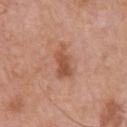Part of a total-body skin-imaging series; this lesion was reviewed on a skin check and was not flagged for biopsy. The tile uses white-light illumination. A male patient approximately 70 years of age. The lesion is on the front of the torso. A 15 mm close-up extracted from a 3D total-body photography capture.